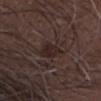<case>
<biopsy_status>not biopsied; imaged during a skin examination</biopsy_status>
<site>head or neck</site>
<lighting>white-light</lighting>
<patient>
  <sex>male</sex>
  <age_approx>75</age_approx>
</patient>
<automated_metrics>
  <eccentricity>0.55</eccentricity>
  <shape_asymmetry>0.25</shape_asymmetry>
  <nevus_likeness_0_100>5</nevus_likeness_0_100>
  <lesion_detection_confidence_0_100>95</lesion_detection_confidence_0_100>
</automated_metrics>
<image>
  <source>total-body photography crop</source>
  <field_of_view_mm>15</field_of_view_mm>
</image>
</case>